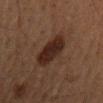notes: total-body-photography surveillance lesion; no biopsy
diameter: about 5.5 mm
anatomic site: the back
image source: total-body-photography crop, ~15 mm field of view
lighting: cross-polarized illumination
subject: male, in their mid- to late 60s